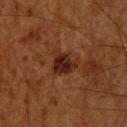imaging modality: total-body-photography crop, ~15 mm field of view
body site: the upper back
lighting: cross-polarized illumination
TBP lesion metrics: a footprint of about 7 mm², a shape eccentricity near 0.45, and a shape-asymmetry score of about 0.35 (0 = symmetric); a border-irregularity rating of about 4/10 and a color-variation rating of about 4/10; an automated nevus-likeness rating near 95 out of 100
diameter: ~3.5 mm (longest diameter)
patient: male, aged around 60